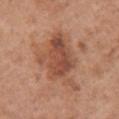Assessment: The lesion was tiled from a total-body skin photograph and was not biopsied. Image and clinical context: The lesion's longest dimension is about 7.5 mm. A 15 mm crop from a total-body photograph taken for skin-cancer surveillance. This is a white-light tile. Located on the chest. A female subject, aged around 65.